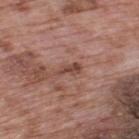{
  "biopsy_status": "not biopsied; imaged during a skin examination",
  "image": {
    "source": "total-body photography crop",
    "field_of_view_mm": 15
  },
  "lesion_size": {
    "long_diameter_mm_approx": 3.0
  },
  "lighting": "white-light",
  "site": "upper back",
  "patient": {
    "sex": "male",
    "age_approx": 70
  }
}